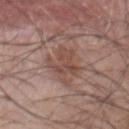<tbp_lesion>
<biopsy_status>not biopsied; imaged during a skin examination</biopsy_status>
<patient>
  <sex>male</sex>
  <age_approx>70</age_approx>
</patient>
<image>
  <source>total-body photography crop</source>
  <field_of_view_mm>15</field_of_view_mm>
</image>
<site>front of the torso</site>
</tbp_lesion>